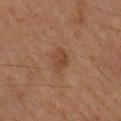notes: catalogued during a skin exam; not biopsied | imaging modality: ~15 mm crop, total-body skin-cancer survey | lighting: cross-polarized | lesion diameter: ≈3 mm | site: the right lower leg | TBP lesion metrics: an area of roughly 4.5 mm² and an outline eccentricity of about 0.7 (0 = round, 1 = elongated); an automated nevus-likeness rating near 35 out of 100 | patient: male, approximately 85 years of age or older.